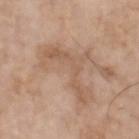- subject · male, approximately 70 years of age
- diameter · about 7.5 mm
- location · the head or neck
- image-analysis metrics · an average lesion color of about L≈57 a*≈18 b*≈30 (CIELAB); a border-irregularity rating of about 10/10, internal color variation of about 2.5 on a 0–10 scale, and peripheral color asymmetry of about 1; an automated nevus-likeness rating near 0 out of 100 and a lesion-detection confidence of about 95/100
- imaging modality · 15 mm crop, total-body photography
- tile lighting · white-light